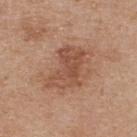<tbp_lesion>
  <biopsy_status>not biopsied; imaged during a skin examination</biopsy_status>
</tbp_lesion>